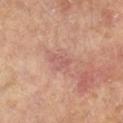  biopsy_status: not biopsied; imaged during a skin examination
  patient:
    sex: female
    age_approx: 75
  site: left lower leg
  lesion_size:
    long_diameter_mm_approx: 2.5
  image:
    source: total-body photography crop
    field_of_view_mm: 15